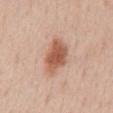{
  "biopsy_status": "not biopsied; imaged during a skin examination",
  "lighting": "white-light",
  "image": {
    "source": "total-body photography crop",
    "field_of_view_mm": 15
  },
  "patient": {
    "sex": "male",
    "age_approx": 55
  },
  "lesion_size": {
    "long_diameter_mm_approx": 5.0
  },
  "automated_metrics": {
    "vs_skin_contrast_norm": 9.0,
    "border_irregularity_0_10": 2.5,
    "peripheral_color_asymmetry": 1.0
  },
  "site": "chest"
}